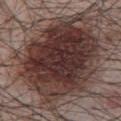Context:
Captured under white-light illumination. Measured at roughly 10 mm in maximum diameter. The subject is a male aged approximately 65. A 15 mm close-up extracted from a 3D total-body photography capture. The total-body-photography lesion software estimated an average lesion color of about L≈33 a*≈18 b*≈19 (CIELAB) and a lesion-to-skin contrast of about 12.5 (normalized; higher = more distinct). And it measured a border-irregularity rating of about 3/10 and peripheral color asymmetry of about 2. It also reported a nevus-likeness score of about 70/100 and a detector confidence of about 100 out of 100 that the crop contains a lesion. The lesion is on the chest.